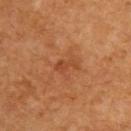Recorded during total-body skin imaging; not selected for excision or biopsy.
A roughly 15 mm field-of-view crop from a total-body skin photograph.
On the upper back.
Imaged with cross-polarized lighting.
The subject is a male aged around 60.